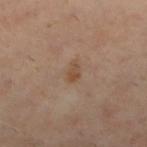Q: Was this lesion biopsied?
A: catalogued during a skin exam; not biopsied
Q: What are the patient's age and sex?
A: female, aged 58–62
Q: How was this image acquired?
A: ~15 mm tile from a whole-body skin photo
Q: How was the tile lit?
A: cross-polarized
Q: Lesion size?
A: ~2.5 mm (longest diameter)
Q: Lesion location?
A: the right leg